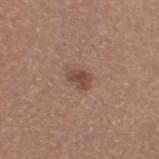Acquisition and patient details:
The lesion is located on the left thigh. A female subject, aged 38 to 42. This image is a 15 mm lesion crop taken from a total-body photograph.